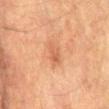biopsy status = imaged on a skin check; not biopsied
image = total-body-photography crop, ~15 mm field of view
location = the mid back
automated lesion analysis = an area of roughly 4 mm², a shape eccentricity near 0.85, and two-axis asymmetry of about 0.3; a lesion color around L≈50 a*≈22 b*≈32 in CIELAB, a lesion–skin lightness drop of about 7, and a normalized lesion–skin contrast near 5.5; a border-irregularity index near 3/10 and a color-variation rating of about 2/10; a detector confidence of about 100 out of 100 that the crop contains a lesion
patient = male, aged 73 to 77
lighting = cross-polarized illumination
diameter = about 3 mm